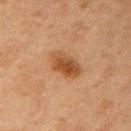Assessment:
No biopsy was performed on this lesion — it was imaged during a full skin examination and was not determined to be concerning.
Clinical summary:
A female subject, aged 43–47. Located on the right upper arm. An algorithmic analysis of the crop reported a classifier nevus-likeness of about 95/100 and a detector confidence of about 100 out of 100 that the crop contains a lesion. The tile uses cross-polarized illumination. The recorded lesion diameter is about 4 mm. Cropped from a total-body skin-imaging series; the visible field is about 15 mm.